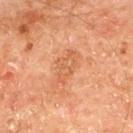About 4 mm across. Located on the mid back. A roughly 15 mm field-of-view crop from a total-body skin photograph. A male subject aged around 65.